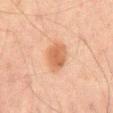Impression: This lesion was catalogued during total-body skin photography and was not selected for biopsy. Clinical summary: A close-up tile cropped from a whole-body skin photograph, about 15 mm across. The recorded lesion diameter is about 4 mm. The lesion is located on the back. The tile uses cross-polarized illumination. The patient is a male aged approximately 65.